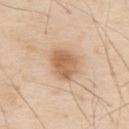Impression: The lesion was photographed on a routine skin check and not biopsied; there is no pathology result. Acquisition and patient details: Located on the upper back. A 15 mm close-up extracted from a 3D total-body photography capture. Captured under white-light illumination. A male patient, aged around 80. Automated tile analysis of the lesion measured a shape eccentricity near 0.55 and a shape-asymmetry score of about 0.2 (0 = symmetric). And it measured a mean CIELAB color near L≈62 a*≈20 b*≈35, about 12 CIELAB-L* units darker than the surrounding skin, and a normalized border contrast of about 7.5. The software also gave a classifier nevus-likeness of about 75/100 and lesion-presence confidence of about 100/100.